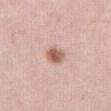Recorded during total-body skin imaging; not selected for excision or biopsy.
Imaged with white-light lighting.
The recorded lesion diameter is about 3 mm.
The patient is a female aged 48–52.
Located on the leg.
A 15 mm close-up tile from a total-body photography series done for melanoma screening.